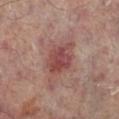Captured during whole-body skin photography for melanoma surveillance; the lesion was not biopsied.
The tile uses cross-polarized illumination.
A 15 mm close-up extracted from a 3D total-body photography capture.
From the right lower leg.
A male patient aged 63 to 67.